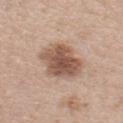Imaged during a routine full-body skin examination; the lesion was not biopsied and no histopathology is available. The lesion is on the chest. A 15 mm close-up tile from a total-body photography series done for melanoma screening. The lesion's longest dimension is about 6 mm. The patient is a female roughly 40 years of age.